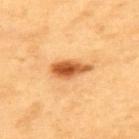| key | value |
|---|---|
| follow-up | imaged on a skin check; not biopsied |
| anatomic site | the upper back |
| image | ~15 mm tile from a whole-body skin photo |
| patient | male, roughly 60 years of age |
| illumination | cross-polarized |